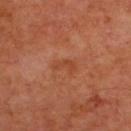{
  "biopsy_status": "not biopsied; imaged during a skin examination",
  "image": {
    "source": "total-body photography crop",
    "field_of_view_mm": 15
  },
  "patient": {
    "sex": "male",
    "age_approx": 70
  },
  "lighting": "cross-polarized",
  "site": "upper back"
}